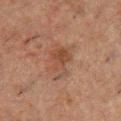{
  "lesion_size": {
    "long_diameter_mm_approx": 4.5
  },
  "patient": {
    "sex": "male",
    "age_approx": 50
  },
  "site": "chest",
  "automated_metrics": {
    "cielab_L": 36,
    "cielab_a": 17,
    "cielab_b": 25,
    "vs_skin_darker_L": 6.0,
    "nevus_likeness_0_100": 5,
    "lesion_detection_confidence_0_100": 100
  },
  "image": {
    "source": "total-body photography crop",
    "field_of_view_mm": 15
  },
  "lighting": "cross-polarized"
}